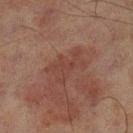workup: no biopsy performed (imaged during a skin exam)
lesion diameter: ≈4.5 mm
lighting: cross-polarized
subject: male, aged 68 to 72
automated metrics: a lesion area of about 8 mm², an eccentricity of roughly 0.85, and a symmetry-axis asymmetry near 0.55; border irregularity of about 7 on a 0–10 scale, a color-variation rating of about 2/10, and a peripheral color-asymmetry measure near 0.5; an automated nevus-likeness rating near 0 out of 100 and a detector confidence of about 100 out of 100 that the crop contains a lesion
body site: the left lower leg
image source: total-body-photography crop, ~15 mm field of view